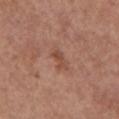Captured during whole-body skin photography for melanoma surveillance; the lesion was not biopsied. A roughly 15 mm field-of-view crop from a total-body skin photograph. A female subject aged around 65. On the left upper arm.